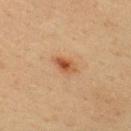No biopsy was performed on this lesion — it was imaged during a full skin examination and was not determined to be concerning.
A 15 mm close-up extracted from a 3D total-body photography capture.
The lesion is located on the upper back.
A male subject, aged approximately 40.
The tile uses cross-polarized illumination.
Measured at roughly 3.5 mm in maximum diameter.